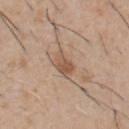follow-up: catalogued during a skin exam; not biopsied | size: about 3 mm | acquisition: ~15 mm tile from a whole-body skin photo | subject: male, roughly 60 years of age | automated lesion analysis: a lesion area of about 4.5 mm²; a lesion color around L≈54 a*≈18 b*≈30 in CIELAB, about 9 CIELAB-L* units darker than the surrounding skin, and a lesion-to-skin contrast of about 7 (normalized; higher = more distinct); an automated nevus-likeness rating near 35 out of 100 and a lesion-detection confidence of about 100/100 | anatomic site: the front of the torso | tile lighting: white-light.